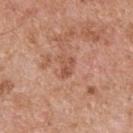notes=total-body-photography surveillance lesion; no biopsy
image=15 mm crop, total-body photography
patient=male, in their mid-50s
site=the upper back
automated metrics=an average lesion color of about L≈53 a*≈24 b*≈31 (CIELAB), about 8 CIELAB-L* units darker than the surrounding skin, and a normalized lesion–skin contrast near 6; a within-lesion color-variation index near 2.5/10 and a peripheral color-asymmetry measure near 1